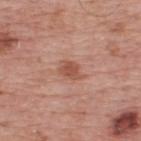Notes:
– workup · total-body-photography surveillance lesion; no biopsy
– automated lesion analysis · an area of roughly 4 mm²
– subject · male, approximately 75 years of age
– body site · the upper back
– illumination · white-light
– image · ~15 mm crop, total-body skin-cancer survey
– size · ~2.5 mm (longest diameter)